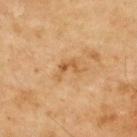Impression: Captured during whole-body skin photography for melanoma surveillance; the lesion was not biopsied. Acquisition and patient details: The lesion is located on the upper back. The total-body-photography lesion software estimated an area of roughly 4 mm², an eccentricity of roughly 0.8, and a symmetry-axis asymmetry near 0.5. The subject is a male aged around 70. A lesion tile, about 15 mm wide, cut from a 3D total-body photograph. The recorded lesion diameter is about 3 mm.